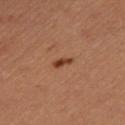Q: Was this lesion biopsied?
A: catalogued during a skin exam; not biopsied
Q: Who is the patient?
A: female, aged around 30
Q: Lesion location?
A: the right thigh
Q: What is the imaging modality?
A: total-body-photography crop, ~15 mm field of view